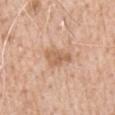Assessment: No biopsy was performed on this lesion — it was imaged during a full skin examination and was not determined to be concerning. Clinical summary: Cropped from a total-body skin-imaging series; the visible field is about 15 mm. Located on the right upper arm. The subject is a male in their 60s. Automated tile analysis of the lesion measured a lesion area of about 6 mm², an eccentricity of roughly 0.8, and a symmetry-axis asymmetry near 0.35. It also reported border irregularity of about 3.5 on a 0–10 scale, a color-variation rating of about 2.5/10, and radial color variation of about 1.